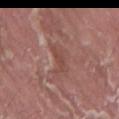The lesion is located on the mid back.
A 15 mm crop from a total-body photograph taken for skin-cancer surveillance.
Longest diameter approximately 3 mm.
A male subject in their 40s.
This is a white-light tile.
Automated tile analysis of the lesion measured an average lesion color of about L≈46 a*≈23 b*≈24 (CIELAB) and a lesion-to-skin contrast of about 5.5 (normalized; higher = more distinct). It also reported a border-irregularity rating of about 5.5/10, a color-variation rating of about 1/10, and peripheral color asymmetry of about 0. The analysis additionally found a detector confidence of about 80 out of 100 that the crop contains a lesion.